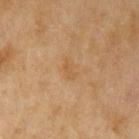notes — catalogued during a skin exam; not biopsied
site — the arm
acquisition — ~15 mm crop, total-body skin-cancer survey
lesion size — ~2.5 mm (longest diameter)
automated lesion analysis — an area of roughly 3 mm², a shape eccentricity near 0.85, and two-axis asymmetry of about 0.2; an average lesion color of about L≈51 a*≈17 b*≈36 (CIELAB), roughly 5 lightness units darker than nearby skin, and a lesion-to-skin contrast of about 4.5 (normalized; higher = more distinct); border irregularity of about 2 on a 0–10 scale and radial color variation of about 0.5
illumination — cross-polarized
subject — female, aged 58 to 62